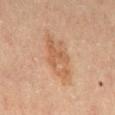notes: no biopsy performed (imaged during a skin exam); image: 15 mm crop, total-body photography; site: the back; patient: male, about 45 years old; image-analysis metrics: a border-irregularity index near 3/10, a within-lesion color-variation index near 4.5/10, and peripheral color asymmetry of about 1.5.